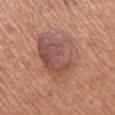Impression: The lesion was photographed on a routine skin check and not biopsied; there is no pathology result. Context: A 15 mm close-up extracted from a 3D total-body photography capture. The subject is a female approximately 60 years of age. On the right upper arm. The tile uses white-light illumination. Longest diameter approximately 8 mm.